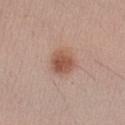Q: Was a biopsy performed?
A: catalogued during a skin exam; not biopsied
Q: Where on the body is the lesion?
A: the left upper arm
Q: Automated lesion metrics?
A: a mean CIELAB color near L≈54 a*≈21 b*≈28 and about 11 CIELAB-L* units darker than the surrounding skin
Q: How was the tile lit?
A: white-light
Q: How was this image acquired?
A: ~15 mm tile from a whole-body skin photo
Q: Who is the patient?
A: male, about 35 years old
Q: How large is the lesion?
A: about 3.5 mm